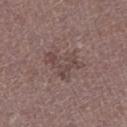workup — imaged on a skin check; not biopsied
lighting — white-light
automated lesion analysis — a lesion–skin lightness drop of about 7 and a normalized border contrast of about 5.5; a nevus-likeness score of about 0/100 and a detector confidence of about 100 out of 100 that the crop contains a lesion
image source — total-body-photography crop, ~15 mm field of view
subject — male, in their mid- to late 70s
anatomic site — the right lower leg